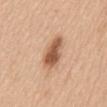biopsy_status: not biopsied; imaged during a skin examination
patient:
  sex: female
  age_approx: 65
lesion_size:
  long_diameter_mm_approx: 4.5
site: mid back
lighting: white-light
image:
  source: total-body photography crop
  field_of_view_mm: 15